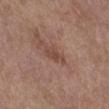workup: no biopsy performed (imaged during a skin exam) | patient: female, in their mid-60s | lesion size: about 3 mm | automated lesion analysis: a lesion area of about 3 mm² and two-axis asymmetry of about 0.35; a border-irregularity index near 4/10 and a peripheral color-asymmetry measure near 0.5 | anatomic site: the right lower leg | image: ~15 mm tile from a whole-body skin photo.